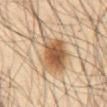Assessment: The lesion was tiled from a total-body skin photograph and was not biopsied. Background: The lesion is on the front of the torso. The patient is a male aged 63 to 67. Captured under cross-polarized illumination. A 15 mm close-up tile from a total-body photography series done for melanoma screening.